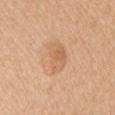Q: Is there a histopathology result?
A: catalogued during a skin exam; not biopsied
Q: What is the imaging modality?
A: 15 mm crop, total-body photography
Q: What lighting was used for the tile?
A: white-light
Q: Where on the body is the lesion?
A: the right upper arm
Q: What are the patient's age and sex?
A: female, aged around 35